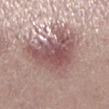Captured during whole-body skin photography for melanoma surveillance; the lesion was not biopsied. Longest diameter approximately 8 mm. Imaged with white-light lighting. A 15 mm crop from a total-body photograph taken for skin-cancer surveillance. The subject is a female approximately 20 years of age. The lesion is located on the left lower leg.